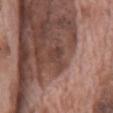Recorded during total-body skin imaging; not selected for excision or biopsy. Located on the mid back. A male patient aged 68–72. Imaged with white-light lighting. A roughly 15 mm field-of-view crop from a total-body skin photograph.